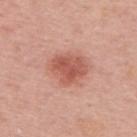Notes:
- workup — no biopsy performed (imaged during a skin exam)
- diameter — ~4.5 mm (longest diameter)
- lighting — white-light illumination
- body site — the upper back
- imaging modality — ~15 mm crop, total-body skin-cancer survey
- patient — male, aged approximately 65